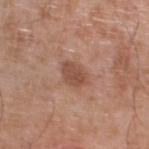Q: Was this lesion biopsied?
A: total-body-photography surveillance lesion; no biopsy
Q: Illumination type?
A: white-light illumination
Q: What is the lesion's diameter?
A: about 3.5 mm
Q: How was this image acquired?
A: total-body-photography crop, ~15 mm field of view
Q: Where on the body is the lesion?
A: the leg
Q: What are the patient's age and sex?
A: male, aged around 80